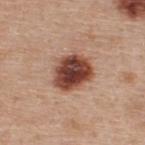Assessment:
Recorded during total-body skin imaging; not selected for excision or biopsy.
Context:
On the upper back. Longest diameter approximately 4.5 mm. This is a white-light tile. A close-up tile cropped from a whole-body skin photograph, about 15 mm across. A male subject, roughly 60 years of age.